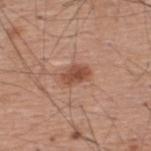<lesion>
  <biopsy_status>not biopsied; imaged during a skin examination</biopsy_status>
</lesion>